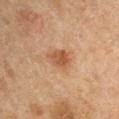Captured during whole-body skin photography for melanoma surveillance; the lesion was not biopsied.
The patient is a male about 50 years old.
The lesion-visualizer software estimated an average lesion color of about L≈41 a*≈18 b*≈29 (CIELAB) and a normalized lesion–skin contrast near 7.5. It also reported a nevus-likeness score of about 75/100.
Imaged with cross-polarized lighting.
Located on the left upper arm.
A roughly 15 mm field-of-view crop from a total-body skin photograph.